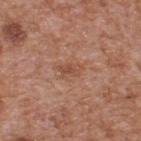Notes:
* subject · male, in their mid-60s
* lighting · white-light illumination
* body site · the back
* imaging modality · ~15 mm tile from a whole-body skin photo
* diameter · ~2.5 mm (longest diameter)